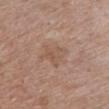Impression:
Imaged during a routine full-body skin examination; the lesion was not biopsied and no histopathology is available.
Image and clinical context:
Imaged with white-light lighting. Measured at roughly 3 mm in maximum diameter. A female subject in their mid-70s. From the chest. A 15 mm close-up extracted from a 3D total-body photography capture.